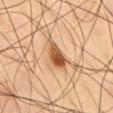Recorded during total-body skin imaging; not selected for excision or biopsy. A male patient aged 58 to 62. The recorded lesion diameter is about 4 mm. Imaged with cross-polarized lighting. A close-up tile cropped from a whole-body skin photograph, about 15 mm across. Located on the abdomen.